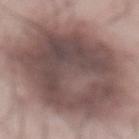biopsy status = imaged on a skin check; not biopsied
location = the front of the torso
image = ~15 mm crop, total-body skin-cancer survey
patient = male, about 55 years old
lesion size = ≈14.5 mm